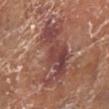follow-up: catalogued during a skin exam; not biopsied
image source: ~15 mm crop, total-body skin-cancer survey
location: the left lower leg
lighting: white-light illumination
automated lesion analysis: an area of roughly 26 mm² and a symmetry-axis asymmetry near 0.4; border irregularity of about 6.5 on a 0–10 scale, a color-variation rating of about 7.5/10, and peripheral color asymmetry of about 2.5
subject: female, aged 73 to 77